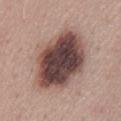Imaged during a routine full-body skin examination; the lesion was not biopsied and no histopathology is available. From the lower back. The subject is a male in their mid- to late 40s. Cropped from a total-body skin-imaging series; the visible field is about 15 mm. The recorded lesion diameter is about 8 mm. This is a white-light tile.